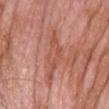About 6 mm across. The total-body-photography lesion software estimated a mean CIELAB color near L≈53 a*≈27 b*≈31 and a lesion–skin lightness drop of about 7. It also reported a border-irregularity index near 6/10 and radial color variation of about 1. It also reported a nevus-likeness score of about 0/100 and lesion-presence confidence of about 55/100. A region of skin cropped from a whole-body photographic capture, roughly 15 mm wide. This is a white-light tile. On the chest. A male patient in their mid- to late 60s.